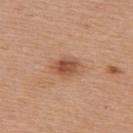patient:
  sex: female
  age_approx: 55
image:
  source: total-body photography crop
  field_of_view_mm: 15
lighting: white-light
site: upper back
lesion_size:
  long_diameter_mm_approx: 3.5